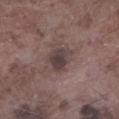– lesion size: ≈3 mm
– subject: male, in their mid- to late 70s
– automated lesion analysis: a lesion area of about 6.5 mm², an outline eccentricity of about 0.55 (0 = round, 1 = elongated), and a shape-asymmetry score of about 0.3 (0 = symmetric); roughly 10 lightness units darker than nearby skin and a normalized border contrast of about 8.5; border irregularity of about 3.5 on a 0–10 scale, a color-variation rating of about 3/10, and a peripheral color-asymmetry measure near 1
– illumination: white-light
– body site: the left lower leg
– image: total-body-photography crop, ~15 mm field of view